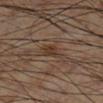Clinical impression: Recorded during total-body skin imaging; not selected for excision or biopsy. Background: A 15 mm close-up extracted from a 3D total-body photography capture. A male subject approximately 55 years of age. Captured under cross-polarized illumination. From the right lower leg.